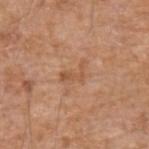{
  "site": "left forearm",
  "image": {
    "source": "total-body photography crop",
    "field_of_view_mm": 15
  },
  "automated_metrics": {
    "area_mm2_approx": 4.0,
    "eccentricity": 0.85,
    "shape_asymmetry": 0.55,
    "cielab_L": 54,
    "cielab_a": 22,
    "cielab_b": 35,
    "vs_skin_darker_L": 7.0,
    "border_irregularity_0_10": 7.0,
    "color_variation_0_10": 1.0,
    "peripheral_color_asymmetry": 0.0,
    "lesion_detection_confidence_0_100": 100
  },
  "patient": {
    "sex": "male",
    "age_approx": 75
  },
  "lighting": "white-light",
  "lesion_size": {
    "long_diameter_mm_approx": 3.5
  }
}